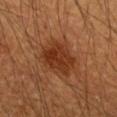Q: Is there a histopathology result?
A: imaged on a skin check; not biopsied
Q: Who is the patient?
A: male, about 55 years old
Q: How large is the lesion?
A: ≈5 mm
Q: Lesion location?
A: the arm
Q: What is the imaging modality?
A: ~15 mm crop, total-body skin-cancer survey
Q: Automated lesion metrics?
A: a shape eccentricity near 0.55; a border-irregularity index near 2.5/10 and a peripheral color-asymmetry measure near 1; a detector confidence of about 100 out of 100 that the crop contains a lesion
Q: What lighting was used for the tile?
A: cross-polarized illumination